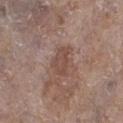Impression:
This lesion was catalogued during total-body skin photography and was not selected for biopsy.
Context:
Located on the left lower leg. A region of skin cropped from a whole-body photographic capture, roughly 15 mm wide. The tile uses white-light illumination. The recorded lesion diameter is about 3.5 mm. A female subject, approximately 85 years of age or older. The lesion-visualizer software estimated a lesion area of about 6.5 mm², a shape eccentricity near 0.8, and two-axis asymmetry of about 0.35. It also reported a border-irregularity index near 3.5/10.